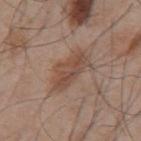Recorded during total-body skin imaging; not selected for excision or biopsy.
From the mid back.
The tile uses white-light illumination.
Cropped from a whole-body photographic skin survey; the tile spans about 15 mm.
A male subject roughly 55 years of age.